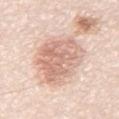Case summary:
- follow-up — no biopsy performed (imaged during a skin exam)
- image — 15 mm crop, total-body photography
- location — the abdomen
- patient — male, roughly 80 years of age